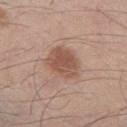biopsy status=total-body-photography surveillance lesion; no biopsy | body site=the left thigh | acquisition=~15 mm tile from a whole-body skin photo | illumination=white-light illumination | lesion diameter=about 5 mm | patient=male, in their mid-40s.